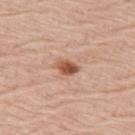Recorded during total-body skin imaging; not selected for excision or biopsy. A lesion tile, about 15 mm wide, cut from a 3D total-body photograph. A female subject roughly 65 years of age. The lesion is on the back. Imaged with white-light lighting. Automated image analysis of the tile measured a mean CIELAB color near L≈54 a*≈24 b*≈32 and about 15 CIELAB-L* units darker than the surrounding skin.